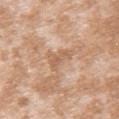biopsy_status: not biopsied; imaged during a skin examination
site: upper back
image:
  source: total-body photography crop
  field_of_view_mm: 15
lighting: white-light
automated_metrics:
  eccentricity: 0.9
  shape_asymmetry: 0.45
  cielab_L: 60
  cielab_a: 20
  cielab_b: 33
  vs_skin_darker_L: 8.0
  vs_skin_contrast_norm: 5.5
  nevus_likeness_0_100: 0
  lesion_detection_confidence_0_100: 95
lesion_size:
  long_diameter_mm_approx: 3.5
patient:
  sex: female
  age_approx: 25